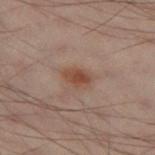body site: the left thigh | lighting: cross-polarized | image: ~15 mm crop, total-body skin-cancer survey | size: ~3 mm (longest diameter) | subject: male, aged 48 to 52.